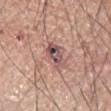Clinical impression:
Part of a total-body skin-imaging series; this lesion was reviewed on a skin check and was not flagged for biopsy.
Background:
The lesion is on the arm. The lesion-visualizer software estimated an average lesion color of about L≈52 a*≈21 b*≈20 (CIELAB) and a lesion–skin lightness drop of about 12. It also reported a border-irregularity rating of about 3.5/10, a within-lesion color-variation index near 10/10, and a peripheral color-asymmetry measure near 4. The recorded lesion diameter is about 3.5 mm. A male subject, aged approximately 65. This is a white-light tile. A lesion tile, about 15 mm wide, cut from a 3D total-body photograph.